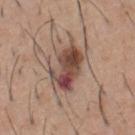Assessment: The lesion was tiled from a total-body skin photograph and was not biopsied. Background: The recorded lesion diameter is about 6 mm. On the chest. A male patient, in their 60s. A lesion tile, about 15 mm wide, cut from a 3D total-body photograph.